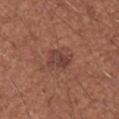Assessment:
Imaged during a routine full-body skin examination; the lesion was not biopsied and no histopathology is available.
Background:
The recorded lesion diameter is about 3 mm. A male patient aged around 55. This is a white-light tile. A roughly 15 mm field-of-view crop from a total-body skin photograph. From the right upper arm.